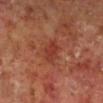notes: total-body-photography surveillance lesion; no biopsy | illumination: cross-polarized | diameter: ≈2.5 mm | automated metrics: a lesion area of about 4.5 mm², an eccentricity of roughly 0.75, and two-axis asymmetry of about 0.25; a normalized border contrast of about 6 | subject: male, roughly 60 years of age | body site: the right lower leg | acquisition: 15 mm crop, total-body photography.